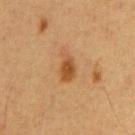follow-up: catalogued during a skin exam; not biopsied
illumination: cross-polarized illumination
acquisition: 15 mm crop, total-body photography
patient: male, approximately 60 years of age
anatomic site: the front of the torso
diameter: ~4 mm (longest diameter)
automated lesion analysis: an area of roughly 6 mm², an eccentricity of roughly 0.85, and two-axis asymmetry of about 0.3; a lesion–skin lightness drop of about 10 and a lesion-to-skin contrast of about 7.5 (normalized; higher = more distinct); border irregularity of about 3.5 on a 0–10 scale, a color-variation rating of about 3.5/10, and peripheral color asymmetry of about 1